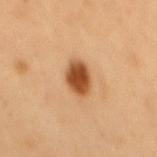notes: total-body-photography surveillance lesion; no biopsy | subject: male, aged approximately 50 | diameter: ≈3.5 mm | automated metrics: an area of roughly 7.5 mm², an outline eccentricity of about 0.7 (0 = round, 1 = elongated), and a shape-asymmetry score of about 0.2 (0 = symmetric); an automated nevus-likeness rating near 100 out of 100 and lesion-presence confidence of about 100/100 | anatomic site: the back | acquisition: total-body-photography crop, ~15 mm field of view | tile lighting: cross-polarized illumination.